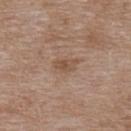- biopsy status — catalogued during a skin exam; not biopsied
- anatomic site — the upper back
- diameter — ≈3 mm
- illumination — white-light
- patient — female, roughly 70 years of age
- acquisition — total-body-photography crop, ~15 mm field of view